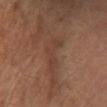Impression: Imaged during a routine full-body skin examination; the lesion was not biopsied and no histopathology is available. Clinical summary: The recorded lesion diameter is about 4.5 mm. The tile uses cross-polarized illumination. A roughly 15 mm field-of-view crop from a total-body skin photograph. On the right lower leg. A female patient in their mid- to late 60s.